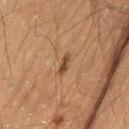Notes:
• notes · total-body-photography surveillance lesion; no biopsy
• subject · male, aged 63–67
• body site · the left thigh
• imaging modality · ~15 mm tile from a whole-body skin photo
• automated lesion analysis · about 9 CIELAB-L* units darker than the surrounding skin and a lesion-to-skin contrast of about 8 (normalized; higher = more distinct)
• tile lighting · cross-polarized illumination